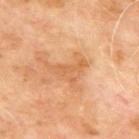<lesion>
  <patient>
    <sex>male</sex>
    <age_approx>65</age_approx>
  </patient>
  <lighting>cross-polarized</lighting>
  <automated_metrics>
    <area_mm2_approx>5.5</area_mm2_approx>
    <eccentricity>0.8</eccentricity>
    <shape_asymmetry>0.6</shape_asymmetry>
    <cielab_L>60</cielab_L>
    <cielab_a>24</cielab_a>
    <cielab_b>41</cielab_b>
    <vs_skin_darker_L>7.0</vs_skin_darker_L>
    <vs_skin_contrast_norm>5.5</vs_skin_contrast_norm>
  </automated_metrics>
  <lesion_size>
    <long_diameter_mm_approx>4.5</long_diameter_mm_approx>
  </lesion_size>
  <image>
    <source>total-body photography crop</source>
    <field_of_view_mm>15</field_of_view_mm>
  </image>
  <site>upper back</site>
</lesion>